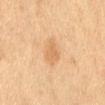Recorded during total-body skin imaging; not selected for excision or biopsy.
On the mid back.
A female patient aged around 55.
An algorithmic analysis of the crop reported a mean CIELAB color near L≈58 a*≈17 b*≈36, a lesion–skin lightness drop of about 7, and a normalized lesion–skin contrast near 5.
A 15 mm crop from a total-body photograph taken for skin-cancer surveillance.
The lesion's longest dimension is about 4 mm.